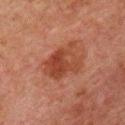The lesion is located on the chest.
The lesion's longest dimension is about 5 mm.
Captured under cross-polarized illumination.
Automated image analysis of the tile measured a lesion color around L≈33 a*≈22 b*≈26 in CIELAB, about 8 CIELAB-L* units darker than the surrounding skin, and a lesion-to-skin contrast of about 7.5 (normalized; higher = more distinct).
The patient is a male in their 60s.
Cropped from a total-body skin-imaging series; the visible field is about 15 mm.